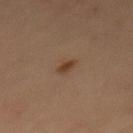notes: catalogued during a skin exam; not biopsied
patient: male, in their mid- to late 30s
lighting: cross-polarized illumination
anatomic site: the mid back
acquisition: ~15 mm crop, total-body skin-cancer survey